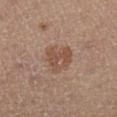notes: no biopsy performed (imaged during a skin exam) | illumination: cross-polarized illumination | acquisition: ~15 mm tile from a whole-body skin photo | site: the left lower leg | patient: female, aged around 45 | size: about 4 mm.